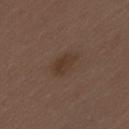Captured during whole-body skin photography for melanoma surveillance; the lesion was not biopsied. Cropped from a whole-body photographic skin survey; the tile spans about 15 mm. From the lower back. A male patient, aged 68–72. Captured under white-light illumination. Automated image analysis of the tile measured a normalized border contrast of about 6.5. It also reported a border-irregularity rating of about 2.5/10 and a within-lesion color-variation index near 2.5/10. The software also gave an automated nevus-likeness rating near 35 out of 100. The recorded lesion diameter is about 3 mm.